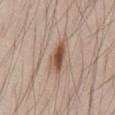workup = imaged on a skin check; not biopsied
illumination = white-light illumination
size = ~4 mm (longest diameter)
location = the back
subject = male, in their mid- to late 30s
image-analysis metrics = a within-lesion color-variation index near 7.5/10
acquisition = ~15 mm crop, total-body skin-cancer survey